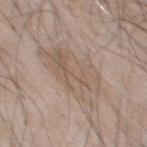No biopsy was performed on this lesion — it was imaged during a full skin examination and was not determined to be concerning.
The subject is a male approximately 65 years of age.
The lesion is located on the front of the torso.
Approximately 7 mm at its widest.
A lesion tile, about 15 mm wide, cut from a 3D total-body photograph.
This is a white-light tile.